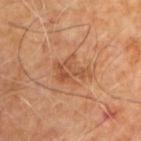Imaged during a routine full-body skin examination; the lesion was not biopsied and no histopathology is available.
The lesion's longest dimension is about 4 mm.
Cropped from a total-body skin-imaging series; the visible field is about 15 mm.
The lesion is located on the upper back.
The tile uses cross-polarized illumination.
A male subject, roughly 60 years of age.